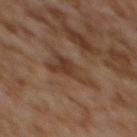Q: Is there a histopathology result?
A: imaged on a skin check; not biopsied
Q: What are the patient's age and sex?
A: female, roughly 60 years of age
Q: What is the lesion's diameter?
A: ~5.5 mm (longest diameter)
Q: Lesion location?
A: the upper back
Q: Illumination type?
A: cross-polarized illumination
Q: What is the imaging modality?
A: ~15 mm tile from a whole-body skin photo